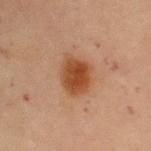An algorithmic analysis of the crop reported an area of roughly 11 mm² and an eccentricity of roughly 0.6. It also reported a lesion color around L≈41 a*≈22 b*≈33 in CIELAB, roughly 11 lightness units darker than nearby skin, and a normalized lesion–skin contrast near 10. Captured under cross-polarized illumination. From the arm. The recorded lesion diameter is about 4 mm. A female subject aged approximately 70. A region of skin cropped from a whole-body photographic capture, roughly 15 mm wide.